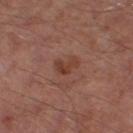The lesion was photographed on a routine skin check and not biopsied; there is no pathology result.
An algorithmic analysis of the crop reported a classifier nevus-likeness of about 20/100.
A male patient aged 63–67.
This image is a 15 mm lesion crop taken from a total-body photograph.
The lesion is on the right thigh.
Longest diameter approximately 3 mm.
This is a cross-polarized tile.